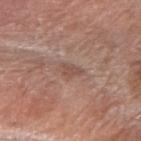anatomic site: the right forearm | subject: female, aged 68 to 72 | imaging modality: ~15 mm tile from a whole-body skin photo | illumination: white-light | size: ~2.5 mm (longest diameter) | TBP lesion metrics: a lesion area of about 3.5 mm², a shape eccentricity near 0.8, and a symmetry-axis asymmetry near 0.25; an average lesion color of about L≈50 a*≈19 b*≈24 (CIELAB), a lesion–skin lightness drop of about 7, and a lesion-to-skin contrast of about 5.5 (normalized; higher = more distinct); internal color variation of about 1 on a 0–10 scale and radial color variation of about 0.5; a classifier nevus-likeness of about 0/100 and a detector confidence of about 100 out of 100 that the crop contains a lesion.